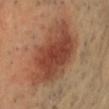biopsy status: no biopsy performed (imaged during a skin exam)
lighting: cross-polarized
lesion size: ≈8.5 mm
location: the head or neck
image: ~15 mm tile from a whole-body skin photo
automated metrics: a lesion color around L≈43 a*≈23 b*≈30 in CIELAB, about 11 CIELAB-L* units darker than the surrounding skin, and a lesion-to-skin contrast of about 8.5 (normalized; higher = more distinct)
subject: male, in their mid- to late 40s